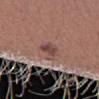<record>
<biopsy_status>not biopsied; imaged during a skin examination</biopsy_status>
<site>right forearm</site>
<patient>
  <sex>female</sex>
  <age_approx>25</age_approx>
</patient>
<image>
  <source>total-body photography crop</source>
  <field_of_view_mm>15</field_of_view_mm>
</image>
</record>